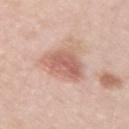The lesion was photographed on a routine skin check and not biopsied; there is no pathology result. A female subject, approximately 40 years of age. The lesion is on the right upper arm. The recorded lesion diameter is about 5 mm. Captured under white-light illumination. A 15 mm crop from a total-body photograph taken for skin-cancer surveillance.